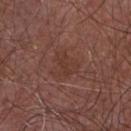• notes · imaged on a skin check; not biopsied
• subject · male, aged 53 to 57
• diameter · ~3.5 mm (longest diameter)
• image · ~15 mm tile from a whole-body skin photo
• illumination · white-light
• site · the front of the torso